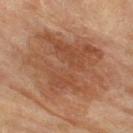workup: imaged on a skin check; not biopsied | image: total-body-photography crop, ~15 mm field of view | patient: female, aged approximately 70 | body site: the left thigh.